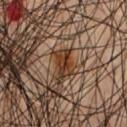Recorded during total-body skin imaging; not selected for excision or biopsy.
A roughly 15 mm field-of-view crop from a total-body skin photograph.
From the chest.
A male patient approximately 45 years of age.
An algorithmic analysis of the crop reported a border-irregularity rating of about 3.5/10, a within-lesion color-variation index near 4.5/10, and radial color variation of about 1.5.